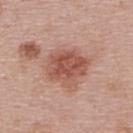No biopsy was performed on this lesion — it was imaged during a full skin examination and was not determined to be concerning.
A female subject aged 48 to 52.
Automated tile analysis of the lesion measured a lesion area of about 16 mm², an outline eccentricity of about 0.55 (0 = round, 1 = elongated), and two-axis asymmetry of about 0.2. The analysis additionally found a mean CIELAB color near L≈53 a*≈24 b*≈28 and a normalized border contrast of about 8. The analysis additionally found a border-irregularity rating of about 2.5/10 and a peripheral color-asymmetry measure near 1.5. It also reported a classifier nevus-likeness of about 70/100 and a detector confidence of about 100 out of 100 that the crop contains a lesion.
A close-up tile cropped from a whole-body skin photograph, about 15 mm across.
The lesion's longest dimension is about 5 mm.
From the upper back.